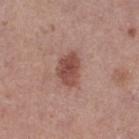Q: Is there a histopathology result?
A: no biopsy performed (imaged during a skin exam)
Q: How was the tile lit?
A: white-light
Q: Patient demographics?
A: female, aged 38–42
Q: Lesion location?
A: the right thigh
Q: How was this image acquired?
A: total-body-photography crop, ~15 mm field of view